The lesion's longest dimension is about 1 mm; a 15 mm crop from a total-body photograph taken for skin-cancer surveillance; the patient is a female aged approximately 50; this is a cross-polarized tile; the lesion is located on the left lower leg:
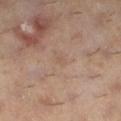On excision, pathology confirmed a seborrheic keratosis.